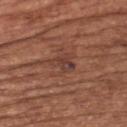Notes:
• biopsy status: total-body-photography surveillance lesion; no biopsy
• tile lighting: white-light
• imaging modality: 15 mm crop, total-body photography
• patient: male, approximately 65 years of age
• anatomic site: the chest